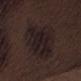Impression:
Captured during whole-body skin photography for melanoma surveillance; the lesion was not biopsied.
Context:
The lesion's longest dimension is about 7.5 mm. A lesion tile, about 15 mm wide, cut from a 3D total-body photograph. An algorithmic analysis of the crop reported an outline eccentricity of about 0.7 (0 = round, 1 = elongated) and a shape-asymmetry score of about 0.25 (0 = symmetric). The analysis additionally found an average lesion color of about L≈18 a*≈11 b*≈11 (CIELAB) and a lesion–skin lightness drop of about 6. The subject is a male aged 68–72. Located on the leg. The tile uses white-light illumination.